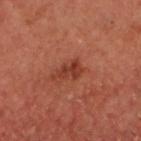Background:
A male patient, roughly 60 years of age. Located on the head or neck. Automated tile analysis of the lesion measured a footprint of about 4 mm² and a symmetry-axis asymmetry near 0.3. The software also gave a nevus-likeness score of about 20/100. Captured under cross-polarized illumination. A roughly 15 mm field-of-view crop from a total-body skin photograph. Longest diameter approximately 2.5 mm.